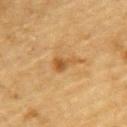follow-up = no biopsy performed (imaged during a skin exam)
automated lesion analysis = a border-irregularity rating of about 4/10 and radial color variation of about 1; lesion-presence confidence of about 100/100
size = ≈3 mm
site = the upper back
image source = total-body-photography crop, ~15 mm field of view
patient = male, aged 83 or over